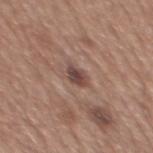| feature | finding |
|---|---|
| workup | catalogued during a skin exam; not biopsied |
| image source | total-body-photography crop, ~15 mm field of view |
| site | the mid back |
| illumination | white-light |
| subject | male, in their mid- to late 60s |
| lesion diameter | ≈3 mm |
| TBP lesion metrics | an average lesion color of about L≈45 a*≈18 b*≈22 (CIELAB), roughly 11 lightness units darker than nearby skin, and a normalized lesion–skin contrast near 8.5; a nevus-likeness score of about 50/100 |